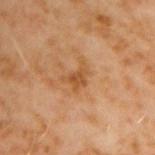Automated image analysis of the tile measured an average lesion color of about L≈49 a*≈21 b*≈38 (CIELAB), a lesion–skin lightness drop of about 8, and a lesion-to-skin contrast of about 6.5 (normalized; higher = more distinct). The software also gave a border-irregularity index near 6/10, internal color variation of about 2.5 on a 0–10 scale, and radial color variation of about 1.
A male subject, about 60 years old.
Approximately 3 mm at its widest.
The tile uses cross-polarized illumination.
A 15 mm close-up extracted from a 3D total-body photography capture.
From the right upper arm.